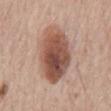biopsy status: no biopsy performed (imaged during a skin exam) | lesion size: ~8 mm (longest diameter) | tile lighting: white-light illumination | subject: male, in their mid-60s | imaging modality: 15 mm crop, total-body photography | TBP lesion metrics: an area of roughly 26 mm², an outline eccentricity of about 0.85 (0 = round, 1 = elongated), and a shape-asymmetry score of about 0.15 (0 = symmetric); a mean CIELAB color near L≈51 a*≈21 b*≈27 and roughly 16 lightness units darker than nearby skin; a border-irregularity rating of about 2/10, a within-lesion color-variation index near 8.5/10, and radial color variation of about 3; a nevus-likeness score of about 100/100 | location: the mid back.